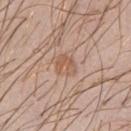{
  "biopsy_status": "not biopsied; imaged during a skin examination",
  "site": "chest",
  "lighting": "white-light",
  "automated_metrics": {
    "shape_asymmetry": 0.25,
    "cielab_L": 57,
    "cielab_a": 19,
    "cielab_b": 30,
    "vs_skin_darker_L": 7.0,
    "vs_skin_contrast_norm": 6.0
  },
  "image": {
    "source": "total-body photography crop",
    "field_of_view_mm": 15
  },
  "lesion_size": {
    "long_diameter_mm_approx": 2.5
  },
  "patient": {
    "sex": "male",
    "age_approx": 40
  }
}